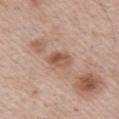Impression: Recorded during total-body skin imaging; not selected for excision or biopsy. Clinical summary: Located on the chest. Captured under white-light illumination. A male patient, aged approximately 65. Cropped from a total-body skin-imaging series; the visible field is about 15 mm. Longest diameter approximately 3 mm. The lesion-visualizer software estimated a lesion color around L≈55 a*≈20 b*≈29 in CIELAB, roughly 10 lightness units darker than nearby skin, and a normalized lesion–skin contrast near 7.5. And it measured an automated nevus-likeness rating near 0 out of 100 and a lesion-detection confidence of about 100/100.